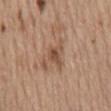Captured during whole-body skin photography for melanoma surveillance; the lesion was not biopsied.